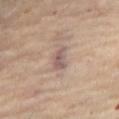Imaged during a routine full-body skin examination; the lesion was not biopsied and no histopathology is available.
A roughly 15 mm field-of-view crop from a total-body skin photograph.
About 2.5 mm across.
A female subject, aged 58–62.
The lesion is located on the front of the torso.
Imaged with cross-polarized lighting.